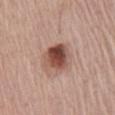Impression: This lesion was catalogued during total-body skin photography and was not selected for biopsy. Acquisition and patient details: The lesion is located on the chest. A 15 mm close-up extracted from a 3D total-body photography capture. The recorded lesion diameter is about 4 mm. Captured under white-light illumination. A male subject roughly 70 years of age.